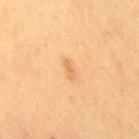The lesion's longest dimension is about 2.5 mm.
A lesion tile, about 15 mm wide, cut from a 3D total-body photograph.
Automated image analysis of the tile measured an eccentricity of roughly 0.9 and a symmetry-axis asymmetry near 0.3. The software also gave a lesion–skin lightness drop of about 7 and a normalized border contrast of about 5. It also reported a lesion-detection confidence of about 100/100.
From the right thigh.
A female subject, in their mid- to late 50s.